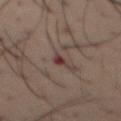lesion_size:
  long_diameter_mm_approx: 2.5
patient:
  sex: male
  age_approx: 40
site: chest
image:
  source: total-body photography crop
  field_of_view_mm: 15
lighting: cross-polarized
automated_metrics:
  shape_asymmetry: 0.45
  nevus_likeness_0_100: 0
  lesion_detection_confidence_0_100: 100